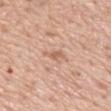Captured during whole-body skin photography for melanoma surveillance; the lesion was not biopsied. The recorded lesion diameter is about 3 mm. This image is a 15 mm lesion crop taken from a total-body photograph. A male patient roughly 60 years of age. On the mid back. Imaged with white-light lighting.